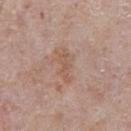Case summary:
• illumination: white-light illumination
• patient: male, in their 60s
• image source: 15 mm crop, total-body photography
• size: ≈4 mm
• site: the abdomen